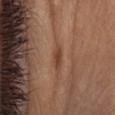* biopsy status — catalogued during a skin exam; not biopsied
* subject — female, in their mid- to late 40s
* TBP lesion metrics — a color-variation rating of about 1.5/10 and a peripheral color-asymmetry measure near 0.5
* image source — 15 mm crop, total-body photography
* body site — the head or neck